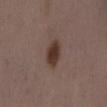Findings:
– workup: total-body-photography surveillance lesion; no biopsy
– lighting: white-light
– lesion diameter: ≈4 mm
– patient: female, aged approximately 35
– anatomic site: the lower back
– imaging modality: total-body-photography crop, ~15 mm field of view
– automated lesion analysis: an area of roughly 6.5 mm² and an eccentricity of roughly 0.85; border irregularity of about 2.5 on a 0–10 scale and internal color variation of about 2.5 on a 0–10 scale; an automated nevus-likeness rating near 100 out of 100